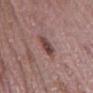Background:
Located on the left thigh. The recorded lesion diameter is about 2.5 mm. A female subject in their 30s. A close-up tile cropped from a whole-body skin photograph, about 15 mm across.